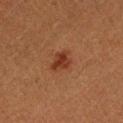Impression:
Captured during whole-body skin photography for melanoma surveillance; the lesion was not biopsied.
Background:
A male patient, approximately 40 years of age. A 15 mm close-up tile from a total-body photography series done for melanoma screening. The lesion is on the left forearm. The tile uses cross-polarized illumination. The lesion's longest dimension is about 3 mm.